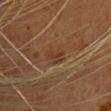Clinical impression:
Imaged during a routine full-body skin examination; the lesion was not biopsied and no histopathology is available.
Background:
Approximately 3 mm at its widest. On the upper back. A female patient, aged 58–62. Cropped from a whole-body photographic skin survey; the tile spans about 15 mm. The tile uses cross-polarized illumination. The lesion-visualizer software estimated two-axis asymmetry of about 0.4. And it measured border irregularity of about 5 on a 0–10 scale and a peripheral color-asymmetry measure near 0.5.